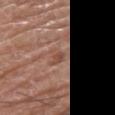Recorded during total-body skin imaging; not selected for excision or biopsy.
Cropped from a total-body skin-imaging series; the visible field is about 15 mm.
The patient is a male about 60 years old.
Captured under white-light illumination.
The lesion's longest dimension is about 3 mm.
The lesion is located on the right thigh.